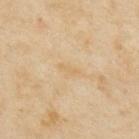Imaged during a routine full-body skin examination; the lesion was not biopsied and no histopathology is available. The patient is a female in their mid-30s. From the back. A close-up tile cropped from a whole-body skin photograph, about 15 mm across.